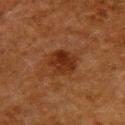The lesion was tiled from a total-body skin photograph and was not biopsied. The lesion is on the upper back. About 3.5 mm across. An algorithmic analysis of the crop reported a lesion area of about 8.5 mm², an eccentricity of roughly 0.5, and two-axis asymmetry of about 0.2. The analysis additionally found about 8 CIELAB-L* units darker than the surrounding skin and a normalized border contrast of about 8.5. The software also gave internal color variation of about 3.5 on a 0–10 scale. It also reported an automated nevus-likeness rating near 65 out of 100 and lesion-presence confidence of about 100/100. Imaged with cross-polarized lighting. A 15 mm crop from a total-body photograph taken for skin-cancer surveillance. A female patient, roughly 50 years of age.